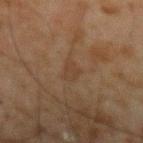Findings:
* workup — imaged on a skin check; not biopsied
* diameter — ~2.5 mm (longest diameter)
* acquisition — ~15 mm crop, total-body skin-cancer survey
* patient — male, about 45 years old
* body site — the left forearm
* automated metrics — a classifier nevus-likeness of about 0/100
* illumination — cross-polarized illumination